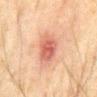Part of a total-body skin-imaging series; this lesion was reviewed on a skin check and was not flagged for biopsy.
The subject is a male in their mid- to late 60s.
An algorithmic analysis of the crop reported a footprint of about 9.5 mm², a shape eccentricity near 0.7, and a symmetry-axis asymmetry near 0.2. The software also gave an average lesion color of about L≈49 a*≈24 b*≈26 (CIELAB), about 10 CIELAB-L* units darker than the surrounding skin, and a lesion-to-skin contrast of about 7.5 (normalized; higher = more distinct). The software also gave a border-irregularity rating of about 2/10, a within-lesion color-variation index near 3.5/10, and radial color variation of about 1.
The tile uses cross-polarized illumination.
A 15 mm crop from a total-body photograph taken for skin-cancer surveillance.
From the abdomen.